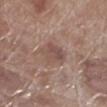Q: Is there a histopathology result?
A: no biopsy performed (imaged during a skin exam)
Q: Illumination type?
A: white-light illumination
Q: Where on the body is the lesion?
A: the left lower leg
Q: What did automated image analysis measure?
A: a mean CIELAB color near L≈50 a*≈18 b*≈22, a lesion–skin lightness drop of about 8, and a normalized lesion–skin contrast near 6
Q: Lesion size?
A: ≈3.5 mm
Q: What are the patient's age and sex?
A: male, approximately 70 years of age
Q: What kind of image is this?
A: ~15 mm tile from a whole-body skin photo